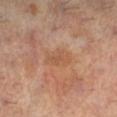Notes:
- follow-up — no biopsy performed (imaged during a skin exam)
- TBP lesion metrics — a mean CIELAB color near L≈56 a*≈22 b*≈34, roughly 6 lightness units darker than nearby skin, and a normalized lesion–skin contrast near 5; border irregularity of about 2.5 on a 0–10 scale, a color-variation rating of about 2.5/10, and peripheral color asymmetry of about 1
- location — the left lower leg
- patient — female, about 60 years old
- acquisition — 15 mm crop, total-body photography